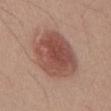{
  "biopsy_status": "not biopsied; imaged during a skin examination",
  "lighting": "white-light",
  "site": "abdomen",
  "patient": {
    "sex": "male",
    "age_approx": 30
  },
  "image": {
    "source": "total-body photography crop",
    "field_of_view_mm": 15
  }
}